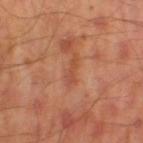Findings:
* follow-up: total-body-photography surveillance lesion; no biopsy
* patient: male, in their mid- to late 40s
* location: the left thigh
* image source: ~15 mm tile from a whole-body skin photo